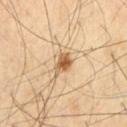Q: Was this lesion biopsied?
A: catalogued during a skin exam; not biopsied
Q: How was the tile lit?
A: cross-polarized
Q: Patient demographics?
A: male, aged 58–62
Q: What is the imaging modality?
A: 15 mm crop, total-body photography
Q: Automated lesion metrics?
A: a footprint of about 5 mm², a shape eccentricity near 0.8, and a symmetry-axis asymmetry near 0.25; an automated nevus-likeness rating near 95 out of 100 and a detector confidence of about 100 out of 100 that the crop contains a lesion
Q: What is the anatomic site?
A: the chest